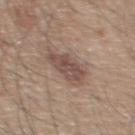biopsy_status: not biopsied; imaged during a skin examination
automated_metrics:
  eccentricity: 0.7
  shape_asymmetry: 0.3
  cielab_L: 49
  cielab_a: 17
  cielab_b: 23
  border_irregularity_0_10: 3.0
  color_variation_0_10: 3.5
  peripheral_color_asymmetry: 1.0
site: back
patient:
  sex: male
  age_approx: 55
lighting: white-light
image:
  source: total-body photography crop
  field_of_view_mm: 15